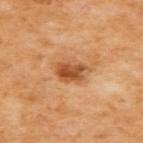notes = no biopsy performed (imaged during a skin exam); lighting = cross-polarized illumination; location = the upper back; subject = female, in their mid- to late 50s; image source = ~15 mm crop, total-body skin-cancer survey; diameter = about 3.5 mm.